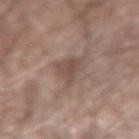Notes:
* follow-up — catalogued during a skin exam; not biopsied
* image source — ~15 mm tile from a whole-body skin photo
* patient — male, in their 60s
* anatomic site — the left forearm